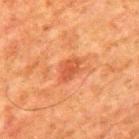Recorded during total-body skin imaging; not selected for excision or biopsy. Imaged with cross-polarized lighting. A close-up tile cropped from a whole-body skin photograph, about 15 mm across. A male subject, about 80 years old. The lesion is on the mid back. Approximately 3 mm at its widest. Automated image analysis of the tile measured a lesion area of about 5 mm², a shape eccentricity near 0.8, and a symmetry-axis asymmetry near 0.25. The analysis additionally found a classifier nevus-likeness of about 25/100 and a detector confidence of about 100 out of 100 that the crop contains a lesion.